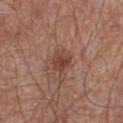<record>
  <biopsy_status>not biopsied; imaged during a skin examination</biopsy_status>
  <image>
    <source>total-body photography crop</source>
    <field_of_view_mm>15</field_of_view_mm>
  </image>
  <patient>
    <sex>male</sex>
    <age_approx>65</age_approx>
  </patient>
  <site>chest</site>
</record>